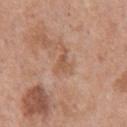{"biopsy_status": "not biopsied; imaged during a skin examination", "image": {"source": "total-body photography crop", "field_of_view_mm": 15}, "site": "front of the torso", "patient": {"sex": "male", "age_approx": 70}}